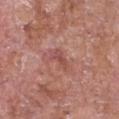<lesion>
  <biopsy_status>not biopsied; imaged during a skin examination</biopsy_status>
  <patient>
    <sex>female</sex>
    <age_approx>75</age_approx>
  </patient>
  <site>chest</site>
  <automated_metrics>
    <area_mm2_approx>3.5</area_mm2_approx>
    <eccentricity>0.85</eccentricity>
    <shape_asymmetry>0.45</shape_asymmetry>
    <color_variation_0_10>1.5</color_variation_0_10>
    <peripheral_color_asymmetry>0.5</peripheral_color_asymmetry>
    <lesion_detection_confidence_0_100>100</lesion_detection_confidence_0_100>
  </automated_metrics>
  <lesion_size>
    <long_diameter_mm_approx>3.0</long_diameter_mm_approx>
  </lesion_size>
  <image>
    <source>total-body photography crop</source>
    <field_of_view_mm>15</field_of_view_mm>
  </image>
  <lighting>white-light</lighting>
</lesion>